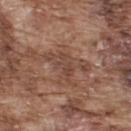Case summary:
• follow-up: imaged on a skin check; not biopsied
• acquisition: ~15 mm crop, total-body skin-cancer survey
• location: the upper back
• illumination: white-light illumination
• lesion size: about 3.5 mm
• subject: male, aged 73–77